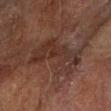{
  "biopsy_status": "not biopsied; imaged during a skin examination",
  "site": "left forearm",
  "patient": {
    "sex": "male",
    "age_approx": 60
  },
  "image": {
    "source": "total-body photography crop",
    "field_of_view_mm": 15
  }
}